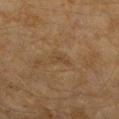Recorded during total-body skin imaging; not selected for excision or biopsy.
The lesion is on the right forearm.
A female subject roughly 60 years of age.
A roughly 15 mm field-of-view crop from a total-body skin photograph.
About 2.5 mm across.
Automated image analysis of the tile measured an area of roughly 3 mm², an eccentricity of roughly 0.85, and a symmetry-axis asymmetry near 0.4. It also reported a border-irregularity index near 4/10, a within-lesion color-variation index near 0/10, and a peripheral color-asymmetry measure near 0. It also reported a nevus-likeness score of about 0/100 and a lesion-detection confidence of about 90/100.
This is a cross-polarized tile.